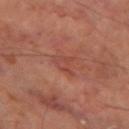The lesion was tiled from a total-body skin photograph and was not biopsied.
This is a cross-polarized tile.
On the left thigh.
Measured at roughly 3 mm in maximum diameter.
The subject is a male aged around 70.
A lesion tile, about 15 mm wide, cut from a 3D total-body photograph.